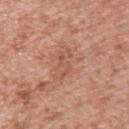{
  "biopsy_status": "not biopsied; imaged during a skin examination",
  "patient": {
    "sex": "male",
    "age_approx": 60
  },
  "site": "upper back",
  "image": {
    "source": "total-body photography crop",
    "field_of_view_mm": 15
  },
  "lighting": "white-light",
  "lesion_size": {
    "long_diameter_mm_approx": 4.0
  },
  "automated_metrics": {
    "area_mm2_approx": 5.0,
    "eccentricity": 0.9,
    "shape_asymmetry": 0.45,
    "cielab_L": 55,
    "cielab_a": 23,
    "cielab_b": 31,
    "vs_skin_darker_L": 7.0,
    "vs_skin_contrast_norm": 5.0
  }
}